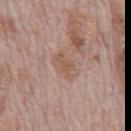Image and clinical context:
A male patient, aged around 70. Cropped from a total-body skin-imaging series; the visible field is about 15 mm. Located on the abdomen.